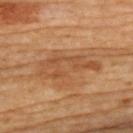| feature | finding |
|---|---|
| biopsy status | catalogued during a skin exam; not biopsied |
| imaging modality | ~15 mm crop, total-body skin-cancer survey |
| patient | female, in their mid-60s |
| lighting | cross-polarized |
| site | the upper back |